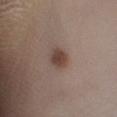Q: Was this lesion biopsied?
A: total-body-photography surveillance lesion; no biopsy
Q: What did automated image analysis measure?
A: an average lesion color of about L≈43 a*≈16 b*≈22 (CIELAB) and about 11 CIELAB-L* units darker than the surrounding skin; border irregularity of about 2 on a 0–10 scale and radial color variation of about 0.5; a detector confidence of about 100 out of 100 that the crop contains a lesion
Q: Where on the body is the lesion?
A: the right lower leg
Q: Patient demographics?
A: female, aged approximately 40
Q: What lighting was used for the tile?
A: white-light illumination
Q: How large is the lesion?
A: about 3 mm
Q: What is the imaging modality?
A: ~15 mm tile from a whole-body skin photo